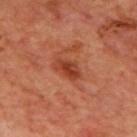| key | value |
|---|---|
| biopsy status | no biopsy performed (imaged during a skin exam) |
| automated metrics | a lesion area of about 5.5 mm², a shape eccentricity near 0.8, and a shape-asymmetry score of about 0.25 (0 = symmetric); a border-irregularity index near 2.5/10, a color-variation rating of about 4.5/10, and radial color variation of about 1.5; a nevus-likeness score of about 95/100 |
| subject | male, about 70 years old |
| lesion size | about 3.5 mm |
| location | the upper back |
| image | ~15 mm crop, total-body skin-cancer survey |
| tile lighting | cross-polarized |